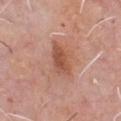biopsy status: catalogued during a skin exam; not biopsied | site: the chest | TBP lesion metrics: roughly 10 lightness units darker than nearby skin and a lesion-to-skin contrast of about 7.5 (normalized; higher = more distinct); a border-irregularity index near 2.5/10 and a color-variation rating of about 3/10 | image source: ~15 mm tile from a whole-body skin photo | lighting: white-light | subject: male, aged around 60.